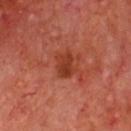biopsy status = imaged on a skin check; not biopsied
image = total-body-photography crop, ~15 mm field of view
subject = male, aged 63–67
lighting = cross-polarized illumination
lesion diameter = ≈3 mm
site = the chest